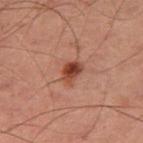{
  "biopsy_status": "not biopsied; imaged during a skin examination",
  "image": {
    "source": "total-body photography crop",
    "field_of_view_mm": 15
  },
  "lighting": "cross-polarized",
  "site": "right thigh",
  "automated_metrics": {
    "cielab_L": 32,
    "cielab_a": 21,
    "cielab_b": 24,
    "vs_skin_contrast_norm": 10.0,
    "border_irregularity_0_10": 3.0,
    "color_variation_0_10": 4.5,
    "peripheral_color_asymmetry": 1.5
  },
  "patient": {
    "sex": "male",
    "age_approx": 60
  }
}